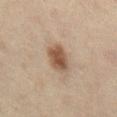Findings:
– follow-up — imaged on a skin check; not biopsied
– acquisition — ~15 mm tile from a whole-body skin photo
– patient — female, roughly 60 years of age
– anatomic site — the lower back